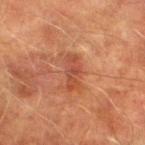biopsy status = no biopsy performed (imaged during a skin exam)
tile lighting = cross-polarized
anatomic site = the leg
acquisition = 15 mm crop, total-body photography
subject = male, about 75 years old